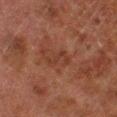Case summary:
– notes: no biopsy performed (imaged during a skin exam)
– patient: male, about 80 years old
– lighting: cross-polarized illumination
– image-analysis metrics: a footprint of about 4.5 mm², a shape eccentricity near 0.85, and two-axis asymmetry of about 0.5; an average lesion color of about L≈29 a*≈19 b*≈24 (CIELAB), about 5 CIELAB-L* units darker than the surrounding skin, and a normalized border contrast of about 5
– anatomic site: the right lower leg
– size: ~3.5 mm (longest diameter)
– image source: ~15 mm crop, total-body skin-cancer survey